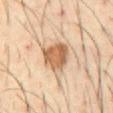The lesion was tiled from a total-body skin photograph and was not biopsied. Automated tile analysis of the lesion measured a shape eccentricity near 0.5 and a shape-asymmetry score of about 0.25 (0 = symmetric). It also reported a classifier nevus-likeness of about 85/100 and a lesion-detection confidence of about 100/100. On the front of the torso. The subject is a male aged approximately 40. About 4 mm across. A close-up tile cropped from a whole-body skin photograph, about 15 mm across.